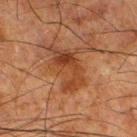<lesion>
  <biopsy_status>not biopsied; imaged during a skin examination</biopsy_status>
  <lighting>cross-polarized</lighting>
  <patient>
    <sex>male</sex>
    <age_approx>80</age_approx>
  </patient>
  <site>right thigh</site>
  <image>
    <source>total-body photography crop</source>
    <field_of_view_mm>15</field_of_view_mm>
  </image>
  <lesion_size>
    <long_diameter_mm_approx>5.5</long_diameter_mm_approx>
  </lesion_size>
</lesion>